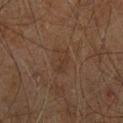follow-up = no biopsy performed (imaged during a skin exam)
subject = male, aged approximately 60
image = 15 mm crop, total-body photography
image-analysis metrics = a footprint of about 5 mm², an eccentricity of roughly 0.75, and a symmetry-axis asymmetry near 0.35; about 4 CIELAB-L* units darker than the surrounding skin and a normalized border contrast of about 4.5; a border-irregularity rating of about 3.5/10, a within-lesion color-variation index near 2.5/10, and peripheral color asymmetry of about 1
body site = the right leg